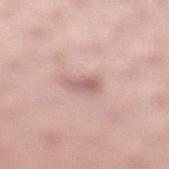Q: Was this lesion biopsied?
A: total-body-photography surveillance lesion; no biopsy
Q: Illumination type?
A: white-light
Q: What are the patient's age and sex?
A: female, aged 23–27
Q: Lesion location?
A: the left lower leg
Q: What is the lesion's diameter?
A: ≈2.5 mm
Q: What kind of image is this?
A: total-body-photography crop, ~15 mm field of view